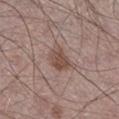Q: Was this lesion biopsied?
A: imaged on a skin check; not biopsied
Q: What is the lesion's diameter?
A: ~3 mm (longest diameter)
Q: What are the patient's age and sex?
A: male, in their mid- to late 60s
Q: What kind of image is this?
A: 15 mm crop, total-body photography
Q: How was the tile lit?
A: white-light
Q: What did automated image analysis measure?
A: a lesion color around L≈48 a*≈18 b*≈23 in CIELAB, a lesion–skin lightness drop of about 10, and a lesion-to-skin contrast of about 7.5 (normalized; higher = more distinct); a border-irregularity rating of about 2.5/10, a within-lesion color-variation index near 2.5/10, and radial color variation of about 1
Q: Lesion location?
A: the right lower leg